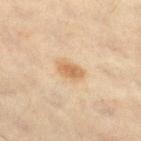Clinical impression:
Recorded during total-body skin imaging; not selected for excision or biopsy.
Image and clinical context:
An algorithmic analysis of the crop reported a mean CIELAB color near L≈61 a*≈17 b*≈36, a lesion–skin lightness drop of about 10, and a lesion-to-skin contrast of about 7.5 (normalized; higher = more distinct). The analysis additionally found border irregularity of about 2 on a 0–10 scale and a color-variation rating of about 2.5/10. A 15 mm close-up extracted from a 3D total-body photography capture. The subject is a male aged 58 to 62. From the right thigh. Imaged with cross-polarized lighting.